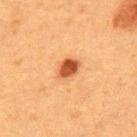Findings:
* workup — total-body-photography surveillance lesion; no biopsy
* tile lighting — cross-polarized illumination
* site — the upper back
* acquisition — ~15 mm tile from a whole-body skin photo
* patient — female, roughly 40 years of age
* diameter — ≈3 mm